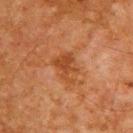This lesion was catalogued during total-body skin photography and was not selected for biopsy.
On the upper back.
Cropped from a whole-body photographic skin survey; the tile spans about 15 mm.
A male subject, aged around 65.